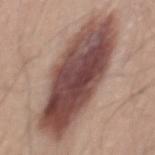Cropped from a total-body skin-imaging series; the visible field is about 15 mm.
Automated image analysis of the tile measured a border-irregularity rating of about 2.5/10 and a peripheral color-asymmetry measure near 3. It also reported an automated nevus-likeness rating near 95 out of 100 and a lesion-detection confidence of about 100/100.
The lesion is on the mid back.
A male subject, roughly 65 years of age.